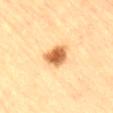Q: Illumination type?
A: cross-polarized
Q: What did automated image analysis measure?
A: a mean CIELAB color near L≈57 a*≈22 b*≈40 and about 17 CIELAB-L* units darker than the surrounding skin
Q: What is the lesion's diameter?
A: ~3 mm (longest diameter)
Q: Where on the body is the lesion?
A: the back
Q: What is the imaging modality?
A: ~15 mm crop, total-body skin-cancer survey
Q: What are the patient's age and sex?
A: male, about 85 years old or older